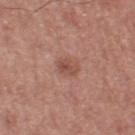follow-up = catalogued during a skin exam; not biopsied | location = the left thigh | acquisition = ~15 mm crop, total-body skin-cancer survey | lesion diameter = ≈2.5 mm | TBP lesion metrics = a lesion color around L≈50 a*≈23 b*≈27 in CIELAB, about 8 CIELAB-L* units darker than the surrounding skin, and a normalized border contrast of about 6 | patient = male, about 70 years old.